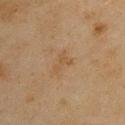Recorded during total-body skin imaging; not selected for excision or biopsy. The recorded lesion diameter is about 3 mm. Cropped from a whole-body photographic skin survey; the tile spans about 15 mm. The tile uses cross-polarized illumination. The lesion is located on the left upper arm. A male patient, roughly 45 years of age.